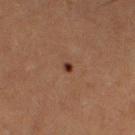{
  "biopsy_status": "not biopsied; imaged during a skin examination",
  "image": {
    "source": "total-body photography crop",
    "field_of_view_mm": 15
  },
  "lighting": "cross-polarized",
  "lesion_size": {
    "long_diameter_mm_approx": 1.0
  },
  "automated_metrics": {
    "eccentricity": 0.5,
    "shape_asymmetry": 0.35,
    "cielab_L": 27,
    "cielab_a": 19,
    "cielab_b": 23,
    "vs_skin_darker_L": 11.0,
    "vs_skin_contrast_norm": 11.5,
    "border_irregularity_0_10": 2.5,
    "color_variation_0_10": 0.0
  },
  "site": "left thigh",
  "patient": {
    "sex": "female",
    "age_approx": 50
  }
}